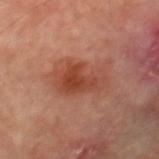{"biopsy_status": "not biopsied; imaged during a skin examination", "site": "leg", "lighting": "cross-polarized", "image": {"source": "total-body photography crop", "field_of_view_mm": 15}, "patient": {"sex": "male", "age_approx": 70}, "lesion_size": {"long_diameter_mm_approx": 5.5}}